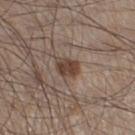Findings:
– notes: no biopsy performed (imaged during a skin exam)
– illumination: white-light illumination
– subject: male, aged around 60
– lesion diameter: ≈2.5 mm
– image: ~15 mm tile from a whole-body skin photo
– location: the left lower leg